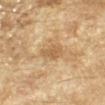workup: imaged on a skin check; not biopsied | site: the left forearm | patient: female, aged approximately 70 | lesion diameter: about 3.5 mm | automated metrics: a footprint of about 5 mm², an outline eccentricity of about 0.75 (0 = round, 1 = elongated), and a symmetry-axis asymmetry near 0.25; a mean CIELAB color near L≈61 a*≈17 b*≈39, a lesion–skin lightness drop of about 9, and a normalized lesion–skin contrast near 6; a within-lesion color-variation index near 1.5/10 and peripheral color asymmetry of about 0.5; lesion-presence confidence of about 95/100 | image source: ~15 mm tile from a whole-body skin photo | tile lighting: cross-polarized illumination.